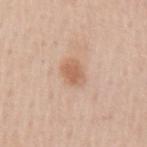Clinical impression:
No biopsy was performed on this lesion — it was imaged during a full skin examination and was not determined to be concerning.
Background:
The tile uses white-light illumination. A region of skin cropped from a whole-body photographic capture, roughly 15 mm wide. From the arm. Measured at roughly 3 mm in maximum diameter. A male subject aged 58 to 62.